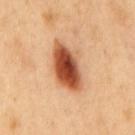The lesion was tiled from a total-body skin photograph and was not biopsied. Captured under cross-polarized illumination. A male patient aged 48–52. The lesion is on the back. A 15 mm crop from a total-body photograph taken for skin-cancer surveillance. Automated tile analysis of the lesion measured a lesion area of about 15 mm², a shape eccentricity near 0.85, and a symmetry-axis asymmetry near 0.2. It also reported a lesion–skin lightness drop of about 20. The analysis additionally found a border-irregularity index near 2.5/10 and a color-variation rating of about 8.5/10. The software also gave a classifier nevus-likeness of about 100/100 and a detector confidence of about 100 out of 100 that the crop contains a lesion. Measured at roughly 6.5 mm in maximum diameter.